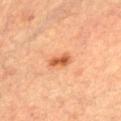Assessment:
Captured during whole-body skin photography for melanoma surveillance; the lesion was not biopsied.
Context:
The tile uses cross-polarized illumination. A female subject, aged 58 to 62. Automated image analysis of the tile measured a footprint of about 4.5 mm², a shape eccentricity near 0.8, and a symmetry-axis asymmetry near 0.2. The analysis additionally found a lesion color around L≈54 a*≈26 b*≈38 in CIELAB, about 11 CIELAB-L* units darker than the surrounding skin, and a normalized border contrast of about 8. It also reported border irregularity of about 2 on a 0–10 scale and radial color variation of about 1. And it measured an automated nevus-likeness rating near 85 out of 100 and lesion-presence confidence of about 100/100. Located on the abdomen. A roughly 15 mm field-of-view crop from a total-body skin photograph. Approximately 3 mm at its widest.